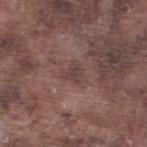  biopsy_status: not biopsied; imaged during a skin examination
  lighting: white-light
  image:
    source: total-body photography crop
    field_of_view_mm: 15
  lesion_size:
    long_diameter_mm_approx: 2.5
  patient:
    sex: male
    age_approx: 75
  site: left lower leg
  automated_metrics:
    eccentricity: 0.55
    shape_asymmetry: 0.45
    vs_skin_contrast_norm: 5.0
    nevus_likeness_0_100: 0
    lesion_detection_confidence_0_100: 100